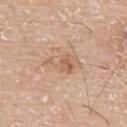Q: Is there a histopathology result?
A: no biopsy performed (imaged during a skin exam)
Q: What is the lesion's diameter?
A: ~5 mm (longest diameter)
Q: Where on the body is the lesion?
A: the back
Q: What kind of image is this?
A: total-body-photography crop, ~15 mm field of view
Q: What lighting was used for the tile?
A: white-light illumination
Q: Who is the patient?
A: male, aged around 75